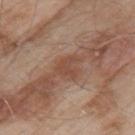- follow-up · imaged on a skin check; not biopsied
- patient · male, aged 53 to 57
- image-analysis metrics · a symmetry-axis asymmetry near 0.5; an average lesion color of about L≈47 a*≈21 b*≈28 (CIELAB), a lesion–skin lightness drop of about 8, and a normalized lesion–skin contrast near 6; border irregularity of about 5.5 on a 0–10 scale and internal color variation of about 1 on a 0–10 scale
- diameter · ≈3 mm
- anatomic site · the left upper arm
- acquisition · ~15 mm tile from a whole-body skin photo
- illumination · white-light illumination